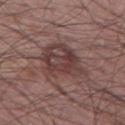Impression:
Imaged during a routine full-body skin examination; the lesion was not biopsied and no histopathology is available.
Context:
Located on the left thigh. A close-up tile cropped from a whole-body skin photograph, about 15 mm across. Captured under white-light illumination. Automated tile analysis of the lesion measured a lesion–skin lightness drop of about 9 and a normalized border contrast of about 7.5. It also reported an automated nevus-likeness rating near 0 out of 100 and a detector confidence of about 95 out of 100 that the crop contains a lesion. Longest diameter approximately 7 mm. A male patient approximately 60 years of age.